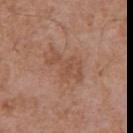Case summary:
• image source — ~15 mm crop, total-body skin-cancer survey
• diameter — ≈5 mm
• location — the chest
• image-analysis metrics — an eccentricity of roughly 0.85 and two-axis asymmetry of about 0.5; a mean CIELAB color near L≈50 a*≈21 b*≈30, about 7 CIELAB-L* units darker than the surrounding skin, and a normalized lesion–skin contrast near 5.5; a nevus-likeness score of about 0/100
• subject — male, in their 70s
• lighting — white-light illumination